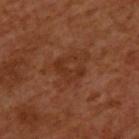Impression:
The lesion was tiled from a total-body skin photograph and was not biopsied.
Clinical summary:
A close-up tile cropped from a whole-body skin photograph, about 15 mm across. Located on the upper back. The lesion-visualizer software estimated a lesion color around L≈32 a*≈23 b*≈31 in CIELAB, a lesion–skin lightness drop of about 6, and a normalized lesion–skin contrast near 6. Approximately 3.5 mm at its widest. A male patient, aged 48 to 52.